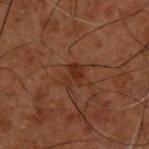notes: total-body-photography surveillance lesion; no biopsy
imaging modality: ~15 mm crop, total-body skin-cancer survey
site: the upper back
automated metrics: a lesion color around L≈22 a*≈18 b*≈23 in CIELAB, roughly 5 lightness units darker than nearby skin, and a lesion-to-skin contrast of about 6.5 (normalized; higher = more distinct); a border-irregularity index near 3.5/10, internal color variation of about 1.5 on a 0–10 scale, and radial color variation of about 0.5
patient: male, roughly 50 years of age
illumination: cross-polarized
lesion diameter: ≈3 mm